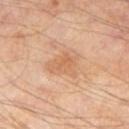This lesion was catalogued during total-body skin photography and was not selected for biopsy. A 15 mm crop from a total-body photograph taken for skin-cancer surveillance. Measured at roughly 4 mm in maximum diameter. The lesion is on the right thigh. The patient is a male in their mid- to late 60s.